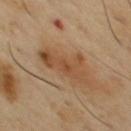Assessment: Captured during whole-body skin photography for melanoma surveillance; the lesion was not biopsied. Acquisition and patient details: About 5.5 mm across. The lesion is located on the chest. Imaged with cross-polarized lighting. A 15 mm crop from a total-body photograph taken for skin-cancer surveillance. The subject is a male aged around 50.